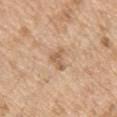The lesion was tiled from a total-body skin photograph and was not biopsied.
The lesion is on the left upper arm.
Imaged with white-light lighting.
The lesion's longest dimension is about 3 mm.
A male subject about 70 years old.
Cropped from a whole-body photographic skin survey; the tile spans about 15 mm.